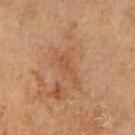Clinical impression: No biopsy was performed on this lesion — it was imaged during a full skin examination and was not determined to be concerning. Background: Captured under cross-polarized illumination. A 15 mm close-up tile from a total-body photography series done for melanoma screening. The total-body-photography lesion software estimated a lesion color around L≈51 a*≈22 b*≈36 in CIELAB and a lesion–skin lightness drop of about 6. The analysis additionally found a within-lesion color-variation index near 0.5/10. The analysis additionally found a classifier nevus-likeness of about 0/100 and lesion-presence confidence of about 100/100. A male patient approximately 70 years of age. On the arm.